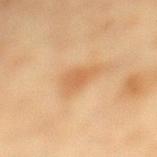Recorded during total-body skin imaging; not selected for excision or biopsy. Captured under cross-polarized illumination. The subject is a female aged 53 to 57. This image is a 15 mm lesion crop taken from a total-body photograph. Located on the left lower leg. Automated image analysis of the tile measured a lesion area of about 5.5 mm². And it measured a lesion–skin lightness drop of about 8 and a normalized lesion–skin contrast near 6. The analysis additionally found a nevus-likeness score of about 70/100.